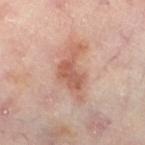Part of a total-body skin-imaging series; this lesion was reviewed on a skin check and was not flagged for biopsy. The lesion is on the left lower leg. Longest diameter approximately 6.5 mm. A 15 mm close-up tile from a total-body photography series done for melanoma screening. The lesion-visualizer software estimated two-axis asymmetry of about 0.55. And it measured border irregularity of about 6.5 on a 0–10 scale, internal color variation of about 4 on a 0–10 scale, and peripheral color asymmetry of about 1. A female subject aged 53 to 57.